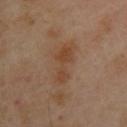Clinical summary: A close-up tile cropped from a whole-body skin photograph, about 15 mm across. The subject is a female aged 38–42. The lesion is located on the upper back.